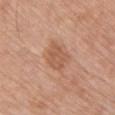Recorded during total-body skin imaging; not selected for excision or biopsy. From the front of the torso. Cropped from a whole-body photographic skin survey; the tile spans about 15 mm. Automated image analysis of the tile measured a footprint of about 7 mm² and an eccentricity of roughly 0.6. The software also gave border irregularity of about 2.5 on a 0–10 scale, internal color variation of about 2.5 on a 0–10 scale, and peripheral color asymmetry of about 1. This is a white-light tile. The patient is a male roughly 70 years of age.